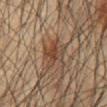| key | value |
|---|---|
| workup | imaged on a skin check; not biopsied |
| imaging modality | total-body-photography crop, ~15 mm field of view |
| illumination | cross-polarized |
| diameter | about 3.5 mm |
| site | the front of the torso |
| subject | male, in their 60s |